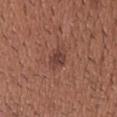location — the head or neck | patient — male, roughly 40 years of age | TBP lesion metrics — a mean CIELAB color near L≈41 a*≈22 b*≈24, a lesion–skin lightness drop of about 8, and a lesion-to-skin contrast of about 7 (normalized; higher = more distinct); internal color variation of about 2 on a 0–10 scale and peripheral color asymmetry of about 0.5 | lesion size — about 2.5 mm | imaging modality — ~15 mm crop, total-body skin-cancer survey.